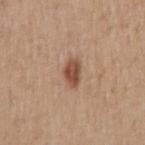  biopsy_status: not biopsied; imaged during a skin examination
  patient:
    sex: male
    age_approx: 55
  site: right upper arm
  lighting: white-light
  lesion_size:
    long_diameter_mm_approx: 3.0
  image:
    source: total-body photography crop
    field_of_view_mm: 15